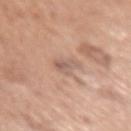Case summary:
– follow-up — no biopsy performed (imaged during a skin exam)
– patient — female, aged 48 to 52
– acquisition — ~15 mm crop, total-body skin-cancer survey
– lighting — white-light
– location — the right upper arm
– automated metrics — a shape eccentricity near 0.85 and two-axis asymmetry of about 0.45; a mean CIELAB color near L≈59 a*≈18 b*≈26, roughly 8 lightness units darker than nearby skin, and a normalized border contrast of about 5.5